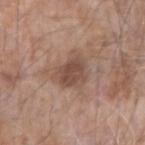Part of a total-body skin-imaging series; this lesion was reviewed on a skin check and was not flagged for biopsy. On the right forearm. A 15 mm close-up tile from a total-body photography series done for melanoma screening. The subject is a male roughly 75 years of age. Imaged with white-light lighting.